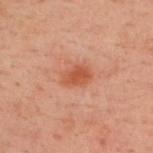Recorded during total-body skin imaging; not selected for excision or biopsy.
A 15 mm crop from a total-body photograph taken for skin-cancer surveillance.
The tile uses cross-polarized illumination.
A male subject aged 38–42.
The total-body-photography lesion software estimated a footprint of about 5.5 mm². The analysis additionally found an average lesion color of about L≈43 a*≈24 b*≈29 (CIELAB), roughly 8 lightness units darker than nearby skin, and a lesion-to-skin contrast of about 7.5 (normalized; higher = more distinct). It also reported a border-irregularity index near 2/10, a within-lesion color-variation index near 2.5/10, and radial color variation of about 1.
The lesion is located on the back.